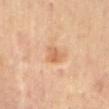Impression:
Part of a total-body skin-imaging series; this lesion was reviewed on a skin check and was not flagged for biopsy.
Background:
This image is a 15 mm lesion crop taken from a total-body photograph. Automated image analysis of the tile measured an area of roughly 4.5 mm² and an outline eccentricity of about 0.6 (0 = round, 1 = elongated). The lesion is on the back. A male patient aged 53 to 57. The lesion's longest dimension is about 3 mm.